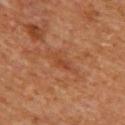notes — total-body-photography surveillance lesion; no biopsy | imaging modality — total-body-photography crop, ~15 mm field of view | body site — the chest | lighting — cross-polarized | diameter — ~3.5 mm (longest diameter) | patient — male, about 60 years old.